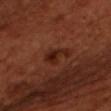<record>
  <lighting>cross-polarized</lighting>
  <automated_metrics>
    <eccentricity>0.85</eccentricity>
    <shape_asymmetry>0.45</shape_asymmetry>
    <cielab_L>23</cielab_L>
    <cielab_a>24</cielab_a>
    <cielab_b>27</cielab_b>
    <vs_skin_darker_L>7.0</vs_skin_darker_L>
    <vs_skin_contrast_norm>8.0</vs_skin_contrast_norm>
    <border_irregularity_0_10>5.0</border_irregularity_0_10>
    <color_variation_0_10>3.5</color_variation_0_10>
    <peripheral_color_asymmetry>1.5</peripheral_color_asymmetry>
    <lesion_detection_confidence_0_100>100</lesion_detection_confidence_0_100>
  </automated_metrics>
  <lesion_size>
    <long_diameter_mm_approx>3.5</long_diameter_mm_approx>
  </lesion_size>
  <patient>
    <sex>male</sex>
    <age_approx>50</age_approx>
  </patient>
  <site>front of the torso</site>
  <image>
    <source>total-body photography crop</source>
    <field_of_view_mm>15</field_of_view_mm>
  </image>
</record>